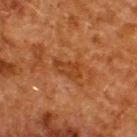Notes:
– biopsy status — total-body-photography surveillance lesion; no biopsy
– lighting — cross-polarized
– image source — total-body-photography crop, ~15 mm field of view
– automated lesion analysis — an eccentricity of roughly 0.85 and a shape-asymmetry score of about 0.3 (0 = symmetric); a mean CIELAB color near L≈31 a*≈22 b*≈32, about 6 CIELAB-L* units darker than the surrounding skin, and a lesion-to-skin contrast of about 6 (normalized; higher = more distinct); an automated nevus-likeness rating near 0 out of 100 and lesion-presence confidence of about 100/100
– body site — the upper back
– patient — male, aged approximately 60